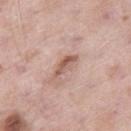notes = catalogued during a skin exam; not biopsied | subject = male, aged 53 to 57 | acquisition = total-body-photography crop, ~15 mm field of view | body site = the right thigh | lighting = white-light.